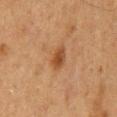Assessment: This lesion was catalogued during total-body skin photography and was not selected for biopsy. Clinical summary: This is a cross-polarized tile. The lesion is located on the mid back. A male subject in their mid- to late 70s. Cropped from a whole-body photographic skin survey; the tile spans about 15 mm. Measured at roughly 3 mm in maximum diameter.